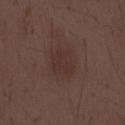follow-up=catalogued during a skin exam; not biopsied
imaging modality=15 mm crop, total-body photography
subject=male, aged 48–52